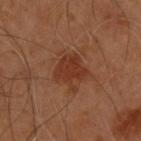<tbp_lesion>
<biopsy_status>not biopsied; imaged during a skin examination</biopsy_status>
<site>upper back</site>
<patient>
  <sex>male</sex>
  <age_approx>55</age_approx>
</patient>
<lighting>cross-polarized</lighting>
<image>
  <source>total-body photography crop</source>
  <field_of_view_mm>15</field_of_view_mm>
</image>
</tbp_lesion>